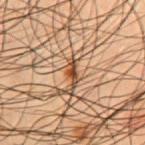Clinical impression: No biopsy was performed on this lesion — it was imaged during a full skin examination and was not determined to be concerning. Clinical summary: The tile uses cross-polarized illumination. On the chest. The patient is a male approximately 55 years of age. Approximately 3 mm at its widest. A 15 mm close-up extracted from a 3D total-body photography capture.